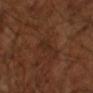Captured during whole-body skin photography for melanoma surveillance; the lesion was not biopsied. Imaged with cross-polarized lighting. The recorded lesion diameter is about 3 mm. A 15 mm crop from a total-body photograph taken for skin-cancer surveillance. Automated tile analysis of the lesion measured a mean CIELAB color near L≈25 a*≈18 b*≈25 and a normalized lesion–skin contrast near 5. And it measured border irregularity of about 3.5 on a 0–10 scale, a color-variation rating of about 0.5/10, and radial color variation of about 0. A male subject, aged 63 to 67.